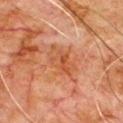No biopsy was performed on this lesion — it was imaged during a full skin examination and was not determined to be concerning. Captured under cross-polarized illumination. A male patient in their 80s. A close-up tile cropped from a whole-body skin photograph, about 15 mm across. The lesion is on the chest. About 4.5 mm across.